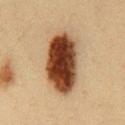Q: Was this lesion biopsied?
A: no biopsy performed (imaged during a skin exam)
Q: How large is the lesion?
A: about 8 mm
Q: Illumination type?
A: cross-polarized illumination
Q: What is the imaging modality?
A: ~15 mm crop, total-body skin-cancer survey
Q: What are the patient's age and sex?
A: male, approximately 35 years of age
Q: Where on the body is the lesion?
A: the front of the torso